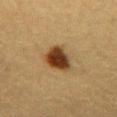notes: imaged on a skin check; not biopsied
body site: the chest
subject: female, aged approximately 55
lighting: cross-polarized
automated lesion analysis: an area of roughly 8.5 mm², an outline eccentricity of about 0.65 (0 = round, 1 = elongated), and a shape-asymmetry score of about 0.2 (0 = symmetric); border irregularity of about 2 on a 0–10 scale, a color-variation rating of about 4.5/10, and peripheral color asymmetry of about 1; a nevus-likeness score of about 100/100 and lesion-presence confidence of about 100/100
size: ~4 mm (longest diameter)
imaging modality: ~15 mm tile from a whole-body skin photo